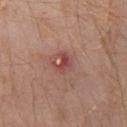Assessment: Captured during whole-body skin photography for melanoma surveillance; the lesion was not biopsied. Background: A close-up tile cropped from a whole-body skin photograph, about 15 mm across. The subject is a male about 30 years old. The lesion is on the left leg.